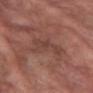• follow-up · imaged on a skin check; not biopsied
• patient · female, approximately 70 years of age
• lesion diameter · ≈4.5 mm
• tile lighting · white-light
• image source · ~15 mm tile from a whole-body skin photo
• location · the arm